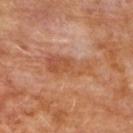Case summary:
- diameter — about 5.5 mm
- image source — total-body-photography crop, ~15 mm field of view
- TBP lesion metrics — a mean CIELAB color near L≈51 a*≈24 b*≈35, about 7 CIELAB-L* units darker than the surrounding skin, and a normalized lesion–skin contrast near 6; an automated nevus-likeness rating near 0 out of 100
- patient — female, roughly 50 years of age
- lighting — cross-polarized
- body site — the upper back